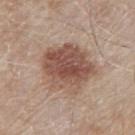workup: total-body-photography surveillance lesion; no biopsy
image source: 15 mm crop, total-body photography
lighting: white-light
lesion diameter: ≈6.5 mm
site: the mid back
automated lesion analysis: an area of roughly 26 mm², an outline eccentricity of about 0.45 (0 = round, 1 = elongated), and two-axis asymmetry of about 0.2; a within-lesion color-variation index near 5.5/10 and peripheral color asymmetry of about 2
subject: male, roughly 80 years of age